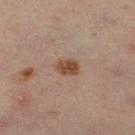| key | value |
|---|---|
| follow-up | no biopsy performed (imaged during a skin exam) |
| site | the leg |
| tile lighting | cross-polarized |
| imaging modality | total-body-photography crop, ~15 mm field of view |
| automated lesion analysis | roughly 11 lightness units darker than nearby skin; a border-irregularity rating of about 2/10 and a within-lesion color-variation index near 3/10 |
| patient | male, aged around 55 |